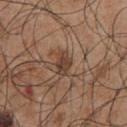{"site": "chest", "patient": {"sex": "male", "age_approx": 55}, "image": {"source": "total-body photography crop", "field_of_view_mm": 15}, "lighting": "white-light", "lesion_size": {"long_diameter_mm_approx": 2.5}}